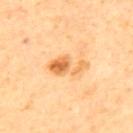A male patient in their 60s. Approximately 5 mm at its widest. The tile uses cross-polarized illumination. A 15 mm crop from a total-body photograph taken for skin-cancer surveillance. The lesion is on the mid back. The lesion-visualizer software estimated lesion-presence confidence of about 100/100.